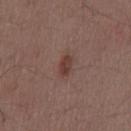notes: no biopsy performed (imaged during a skin exam); lesion size: ~2.5 mm (longest diameter); image source: total-body-photography crop, ~15 mm field of view; illumination: white-light; patient: male, aged 48–52; body site: the lower back.